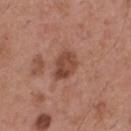A roughly 15 mm field-of-view crop from a total-body skin photograph.
The lesion is located on the mid back.
About 3.5 mm across.
A male subject, aged 53 to 57.
This is a white-light tile.